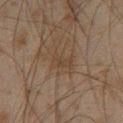The lesion was tiled from a total-body skin photograph and was not biopsied.